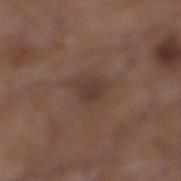The lesion was tiled from a total-body skin photograph and was not biopsied.
On the right lower leg.
The lesion-visualizer software estimated a lesion area of about 3.5 mm² and a symmetry-axis asymmetry near 0.25. The analysis additionally found an automated nevus-likeness rating near 0 out of 100 and a lesion-detection confidence of about 100/100.
A 15 mm crop from a total-body photograph taken for skin-cancer surveillance.
A male subject about 55 years old.